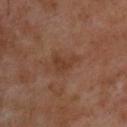  biopsy_status: not biopsied; imaged during a skin examination
  image:
    source: total-body photography crop
    field_of_view_mm: 15
  patient:
    sex: male
    age_approx: 55
  site: upper back